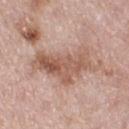workup: no biopsy performed (imaged during a skin exam)
site: the right thigh
image source: ~15 mm crop, total-body skin-cancer survey
subject: female, in their 50s
illumination: white-light illumination